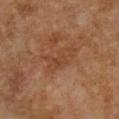Image and clinical context:
A female patient, aged approximately 60. The lesion's longest dimension is about 4.5 mm. A 15 mm crop from a total-body photograph taken for skin-cancer surveillance. Automated image analysis of the tile measured a border-irregularity index near 6/10 and a peripheral color-asymmetry measure near 0.5. The analysis additionally found a nevus-likeness score of about 0/100 and lesion-presence confidence of about 100/100. Imaged with cross-polarized lighting. Located on the front of the torso.